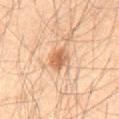This lesion was catalogued during total-body skin photography and was not selected for biopsy.
From the abdomen.
A roughly 15 mm field-of-view crop from a total-body skin photograph.
A male patient aged around 60.
This is a cross-polarized tile.